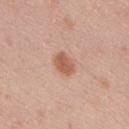follow-up=total-body-photography surveillance lesion; no biopsy | acquisition=total-body-photography crop, ~15 mm field of view | anatomic site=the upper back | subject=male, aged approximately 45 | lesion size=~3 mm (longest diameter).